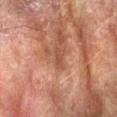Q: Is there a histopathology result?
A: no biopsy performed (imaged during a skin exam)
Q: Automated lesion metrics?
A: an area of roughly 2.5 mm², a shape eccentricity near 0.95, and a symmetry-axis asymmetry near 0.35; a border-irregularity index near 4/10 and radial color variation of about 0
Q: Illumination type?
A: cross-polarized
Q: What is the lesion's diameter?
A: ~2.5 mm (longest diameter)
Q: How was this image acquired?
A: ~15 mm crop, total-body skin-cancer survey
Q: What is the anatomic site?
A: the left forearm
Q: Who is the patient?
A: male, aged around 75